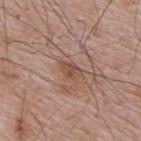biopsy status: catalogued during a skin exam; not biopsied
patient: male, approximately 65 years of age
anatomic site: the back
illumination: white-light
image-analysis metrics: a shape eccentricity near 0.8 and a shape-asymmetry score of about 0.3 (0 = symmetric)
imaging modality: ~15 mm tile from a whole-body skin photo